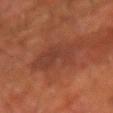Image and clinical context:
A 15 mm crop from a total-body photograph taken for skin-cancer surveillance. A male subject, approximately 60 years of age. From the right forearm.